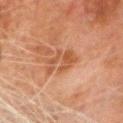workup: catalogued during a skin exam; not biopsied | subject: male, approximately 80 years of age | imaging modality: 15 mm crop, total-body photography | anatomic site: the head or neck.